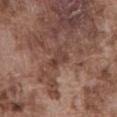<case>
  <biopsy_status>not biopsied; imaged during a skin examination</biopsy_status>
  <site>abdomen</site>
  <automated_metrics>
    <border_irregularity_0_10>4.0</border_irregularity_0_10>
    <color_variation_0_10>1.5</color_variation_0_10>
    <peripheral_color_asymmetry>0.0</peripheral_color_asymmetry>
    <nevus_likeness_0_100>0</nevus_likeness_0_100>
    <lesion_detection_confidence_0_100>90</lesion_detection_confidence_0_100>
  </automated_metrics>
  <patient>
    <sex>male</sex>
    <age_approx>75</age_approx>
  </patient>
  <lighting>white-light</lighting>
  <image>
    <source>total-body photography crop</source>
    <field_of_view_mm>15</field_of_view_mm>
  </image>
</case>